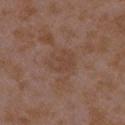Captured during whole-body skin photography for melanoma surveillance; the lesion was not biopsied.
Longest diameter approximately 3.5 mm.
Imaged with white-light lighting.
Cropped from a whole-body photographic skin survey; the tile spans about 15 mm.
From the arm.
Automated tile analysis of the lesion measured a lesion-to-skin contrast of about 4.5 (normalized; higher = more distinct). And it measured a border-irregularity index near 3/10, internal color variation of about 1.5 on a 0–10 scale, and a peripheral color-asymmetry measure near 0.5.
A female patient, about 35 years old.